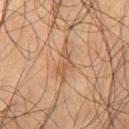{
  "biopsy_status": "not biopsied; imaged during a skin examination",
  "lighting": "cross-polarized",
  "patient": {
    "sex": "male",
    "age_approx": 55
  },
  "image": {
    "source": "total-body photography crop",
    "field_of_view_mm": 15
  },
  "lesion_size": {
    "long_diameter_mm_approx": 4.0
  },
  "site": "right thigh"
}